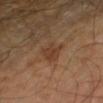No biopsy was performed on this lesion — it was imaged during a full skin examination and was not determined to be concerning. The lesion's longest dimension is about 3 mm. From the left arm. Cropped from a whole-body photographic skin survey; the tile spans about 15 mm. Imaged with cross-polarized lighting. A male patient approximately 60 years of age.